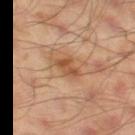notes: catalogued during a skin exam; not biopsied
site: the leg
acquisition: ~15 mm crop, total-body skin-cancer survey
automated metrics: two-axis asymmetry of about 0.35; a lesion color around L≈51 a*≈22 b*≈36 in CIELAB, a lesion–skin lightness drop of about 10, and a lesion-to-skin contrast of about 7.5 (normalized; higher = more distinct); lesion-presence confidence of about 100/100
tile lighting: cross-polarized
subject: male, approximately 45 years of age
size: ≈2.5 mm